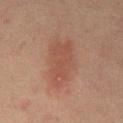| field | value |
|---|---|
| follow-up | catalogued during a skin exam; not biopsied |
| anatomic site | the mid back |
| diameter | ~6.5 mm (longest diameter) |
| patient | male, aged around 65 |
| acquisition | ~15 mm crop, total-body skin-cancer survey |
| illumination | cross-polarized illumination |